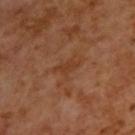Assessment:
Part of a total-body skin-imaging series; this lesion was reviewed on a skin check and was not flagged for biopsy.
Acquisition and patient details:
The lesion is on the upper back. A 15 mm close-up tile from a total-body photography series done for melanoma screening. The lesion's longest dimension is about 3 mm. The lesion-visualizer software estimated a border-irregularity rating of about 4.5/10. And it measured a classifier nevus-likeness of about 0/100 and a detector confidence of about 100 out of 100 that the crop contains a lesion. The patient is a male aged around 65.